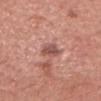No biopsy was performed on this lesion — it was imaged during a full skin examination and was not determined to be concerning. Located on the head or neck. The patient is a female about 65 years old. This is a white-light tile. A roughly 15 mm field-of-view crop from a total-body skin photograph. The total-body-photography lesion software estimated a border-irregularity rating of about 2.5/10, internal color variation of about 4 on a 0–10 scale, and a peripheral color-asymmetry measure near 1.5. Longest diameter approximately 3 mm.